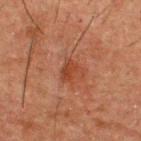Captured during whole-body skin photography for melanoma surveillance; the lesion was not biopsied. A male patient aged 48 to 52. The lesion is located on the upper back. Automated tile analysis of the lesion measured a lesion area of about 4.5 mm² and a shape-asymmetry score of about 0.25 (0 = symmetric). The software also gave an average lesion color of about L≈34 a*≈23 b*≈28 (CIELAB) and a normalized lesion–skin contrast near 6.5. A lesion tile, about 15 mm wide, cut from a 3D total-body photograph.